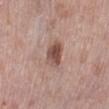The lesion is located on the left lower leg. The tile uses white-light illumination. An algorithmic analysis of the crop reported a symmetry-axis asymmetry near 0.2. The software also gave a lesion color around L≈50 a*≈20 b*≈24 in CIELAB, roughly 13 lightness units darker than nearby skin, and a normalized border contrast of about 9. It also reported border irregularity of about 2 on a 0–10 scale, a color-variation rating of about 4.5/10, and peripheral color asymmetry of about 1.5. The analysis additionally found an automated nevus-likeness rating near 60 out of 100 and a detector confidence of about 100 out of 100 that the crop contains a lesion. Approximately 3.5 mm at its widest. A lesion tile, about 15 mm wide, cut from a 3D total-body photograph. A female patient approximately 65 years of age.